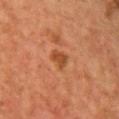<tbp_lesion>
  <biopsy_status>not biopsied; imaged during a skin examination</biopsy_status>
  <site>chest</site>
  <image>
    <source>total-body photography crop</source>
    <field_of_view_mm>15</field_of_view_mm>
  </image>
  <patient>
    <sex>female</sex>
    <age_approx>60</age_approx>
  </patient>
  <lighting>cross-polarized</lighting>
  <lesion_size>
    <long_diameter_mm_approx>2.5</long_diameter_mm_approx>
  </lesion_size>
</tbp_lesion>